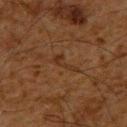Background:
The lesion is on the upper back. Automated tile analysis of the lesion measured an eccentricity of roughly 0.95 and a shape-asymmetry score of about 0.65 (0 = symmetric). It also reported internal color variation of about 0 on a 0–10 scale. The analysis additionally found an automated nevus-likeness rating near 0 out of 100 and a detector confidence of about 95 out of 100 that the crop contains a lesion. A male patient aged 58 to 62. The lesion's longest dimension is about 3.5 mm. A 15 mm close-up extracted from a 3D total-body photography capture. Captured under cross-polarized illumination.